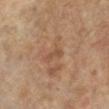A 15 mm close-up extracted from a 3D total-body photography capture.
A female patient, aged 63–67.
On the right leg.
The recorded lesion diameter is about 2.5 mm.